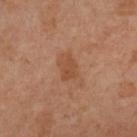Q: Was this lesion biopsied?
A: total-body-photography surveillance lesion; no biopsy
Q: Automated lesion metrics?
A: a lesion area of about 5.5 mm², an outline eccentricity of about 0.75 (0 = round, 1 = elongated), and two-axis asymmetry of about 0.25; a classifier nevus-likeness of about 15/100 and a detector confidence of about 100 out of 100 that the crop contains a lesion
Q: Who is the patient?
A: female, about 50 years old
Q: How was this image acquired?
A: ~15 mm crop, total-body skin-cancer survey
Q: What is the anatomic site?
A: the left lower leg